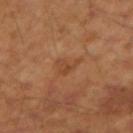Assessment:
The lesion was tiled from a total-body skin photograph and was not biopsied.
Background:
A close-up tile cropped from a whole-body skin photograph, about 15 mm across. A male subject aged around 45. The lesion is located on the right forearm. Approximately 2.5 mm at its widest. Imaged with cross-polarized lighting.